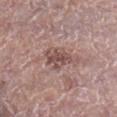The lesion was photographed on a routine skin check and not biopsied; there is no pathology result. A lesion tile, about 15 mm wide, cut from a 3D total-body photograph. On the right lower leg. Imaged with white-light lighting. A male subject approximately 55 years of age. An algorithmic analysis of the crop reported a footprint of about 6.5 mm² and a symmetry-axis asymmetry near 0.3. The software also gave a nevus-likeness score of about 0/100 and a lesion-detection confidence of about 100/100.